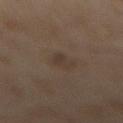Notes:
• follow-up — total-body-photography surveillance lesion; no biopsy
• illumination — cross-polarized illumination
• image — 15 mm crop, total-body photography
• body site — the back
• subject — female, roughly 60 years of age